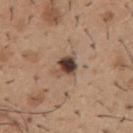{
  "biopsy_status": "not biopsied; imaged during a skin examination",
  "image": {
    "source": "total-body photography crop",
    "field_of_view_mm": 15
  },
  "patient": {
    "sex": "male",
    "age_approx": 40
  },
  "lighting": "white-light",
  "site": "mid back",
  "lesion_size": {
    "long_diameter_mm_approx": 3.0
  }
}